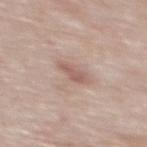follow-up — catalogued during a skin exam; not biopsied | body site — the mid back | image source — 15 mm crop, total-body photography | patient — male, roughly 55 years of age | tile lighting — white-light | lesion size — about 3 mm | automated lesion analysis — a lesion area of about 3.5 mm²; a border-irregularity index near 3.5/10, a color-variation rating of about 1/10, and a peripheral color-asymmetry measure near 0.